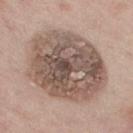A close-up tile cropped from a whole-body skin photograph, about 15 mm across.
From the right thigh.
A male subject, aged 73 to 77.